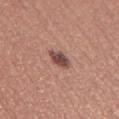Impression: The lesion was photographed on a routine skin check and not biopsied; there is no pathology result. Background: The lesion's longest dimension is about 3 mm. Imaged with white-light lighting. An algorithmic analysis of the crop reported a lesion area of about 4.5 mm² and an eccentricity of roughly 0.75. And it measured a lesion color around L≈47 a*≈22 b*≈23 in CIELAB and a normalized lesion–skin contrast near 10.5. It also reported a border-irregularity rating of about 2.5/10 and radial color variation of about 1. Located on the left thigh. A female subject, about 30 years old. A close-up tile cropped from a whole-body skin photograph, about 15 mm across.